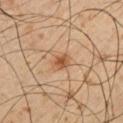Q: Was this lesion biopsied?
A: no biopsy performed (imaged during a skin exam)
Q: How was this image acquired?
A: 15 mm crop, total-body photography
Q: Who is the patient?
A: male, approximately 50 years of age
Q: What is the anatomic site?
A: the left upper arm